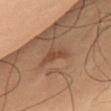Part of a total-body skin-imaging series; this lesion was reviewed on a skin check and was not flagged for biopsy. The lesion is located on the chest. The lesion's longest dimension is about 3 mm. A male subject aged 63–67. Imaged with cross-polarized lighting. A roughly 15 mm field-of-view crop from a total-body skin photograph.